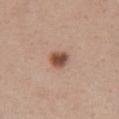This lesion was catalogued during total-body skin photography and was not selected for biopsy. Cropped from a total-body skin-imaging series; the visible field is about 15 mm. Automated tile analysis of the lesion measured a border-irregularity rating of about 1.5/10, internal color variation of about 4.5 on a 0–10 scale, and peripheral color asymmetry of about 1.5. It also reported an automated nevus-likeness rating near 100 out of 100 and a lesion-detection confidence of about 100/100. A female subject approximately 55 years of age. Approximately 2.5 mm at its widest. The lesion is located on the front of the torso.